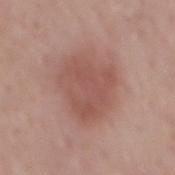{"site": "mid back", "automated_metrics": {"area_mm2_approx": 21.0, "eccentricity": 0.55, "shape_asymmetry": 0.3, "border_irregularity_0_10": 3.5, "color_variation_0_10": 2.5, "peripheral_color_asymmetry": 1.0, "nevus_likeness_0_100": 55, "lesion_detection_confidence_0_100": 100}, "image": {"source": "total-body photography crop", "field_of_view_mm": 15}, "lesion_size": {"long_diameter_mm_approx": 6.0}, "patient": {"sex": "male", "age_approx": 60}, "lighting": "white-light"}